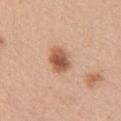<record>
<biopsy_status>not biopsied; imaged during a skin examination</biopsy_status>
<site>left upper arm</site>
<patient>
  <sex>female</sex>
  <age_approx>30</age_approx>
</patient>
<image>
  <source>total-body photography crop</source>
  <field_of_view_mm>15</field_of_view_mm>
</image>
<lesion_size>
  <long_diameter_mm_approx>3.0</long_diameter_mm_approx>
</lesion_size>
<lighting>white-light</lighting>
<automated_metrics>
  <nevus_likeness_0_100>100</nevus_likeness_0_100>
  <lesion_detection_confidence_0_100>100</lesion_detection_confidence_0_100>
</automated_metrics>
</record>